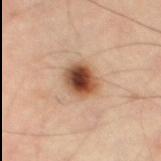notes: catalogued during a skin exam; not biopsied | patient: male, aged approximately 55 | imaging modality: total-body-photography crop, ~15 mm field of view | body site: the leg | tile lighting: cross-polarized illumination | image-analysis metrics: a border-irregularity rating of about 1.5/10 and a color-variation rating of about 9/10; an automated nevus-likeness rating near 100 out of 100 and a lesion-detection confidence of about 100/100.